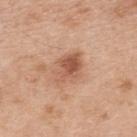biopsy status: imaged on a skin check; not biopsied | anatomic site: the upper back | subject: male, in their 70s | tile lighting: white-light illumination | acquisition: 15 mm crop, total-body photography | diameter: about 4.5 mm.